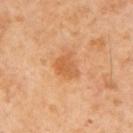Clinical impression:
Part of a total-body skin-imaging series; this lesion was reviewed on a skin check and was not flagged for biopsy.
Image and clinical context:
A 15 mm crop from a total-body photograph taken for skin-cancer surveillance. The patient is a male in their mid- to late 60s. Automated tile analysis of the lesion measured a lesion area of about 6 mm², a shape eccentricity near 0.6, and a shape-asymmetry score of about 0.3 (0 = symmetric). It also reported border irregularity of about 3 on a 0–10 scale, a within-lesion color-variation index near 3/10, and a peripheral color-asymmetry measure near 1. Measured at roughly 3 mm in maximum diameter.